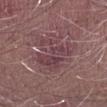Assessment: Imaged during a routine full-body skin examination; the lesion was not biopsied and no histopathology is available. Context: Located on the arm. This is a white-light tile. The lesion's longest dimension is about 7 mm. A 15 mm close-up tile from a total-body photography series done for melanoma screening. The subject is a male in their mid- to late 60s.